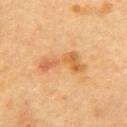Captured during whole-body skin photography for melanoma surveillance; the lesion was not biopsied. The patient is a female aged approximately 60. From the back. Captured under cross-polarized illumination. A lesion tile, about 15 mm wide, cut from a 3D total-body photograph. The recorded lesion diameter is about 5.5 mm.